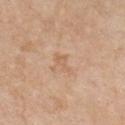{"biopsy_status": "not biopsied; imaged during a skin examination", "automated_metrics": {"area_mm2_approx": 3.0, "eccentricity": 0.85, "shape_asymmetry": 0.4, "cielab_L": 62, "cielab_a": 18, "cielab_b": 34, "vs_skin_darker_L": 6.0, "vs_skin_contrast_norm": 5.0, "nevus_likeness_0_100": 0, "lesion_detection_confidence_0_100": 100}, "image": {"source": "total-body photography crop", "field_of_view_mm": 15}, "site": "front of the torso", "patient": {"sex": "female", "age_approx": 65}, "lesion_size": {"long_diameter_mm_approx": 3.0}, "lighting": "white-light"}